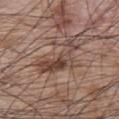Image and clinical context:
The lesion is located on the back. A 15 mm crop from a total-body photograph taken for skin-cancer surveillance. A male patient aged 63–67.